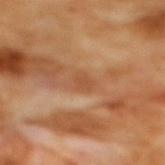Q: Was a biopsy performed?
A: imaged on a skin check; not biopsied
Q: Illumination type?
A: cross-polarized illumination
Q: How large is the lesion?
A: ~2.5 mm (longest diameter)
Q: What kind of image is this?
A: ~15 mm crop, total-body skin-cancer survey
Q: What is the anatomic site?
A: the mid back
Q: What are the patient's age and sex?
A: female, approximately 55 years of age